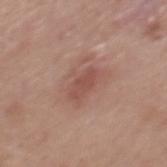A 15 mm crop from a total-body photograph taken for skin-cancer surveillance.
Automated tile analysis of the lesion measured a lesion color around L≈49 a*≈23 b*≈25 in CIELAB, about 8 CIELAB-L* units darker than the surrounding skin, and a normalized lesion–skin contrast near 6. The software also gave a border-irregularity index near 2.5/10, a color-variation rating of about 1/10, and radial color variation of about 0.5. And it measured an automated nevus-likeness rating near 35 out of 100 and lesion-presence confidence of about 100/100.
The lesion is located on the mid back.
A male subject, aged 48 to 52.